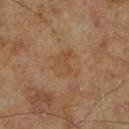The lesion was photographed on a routine skin check and not biopsied; there is no pathology result. Located on the right lower leg. Captured under cross-polarized illumination. The patient is a male about 70 years old. An algorithmic analysis of the crop reported a classifier nevus-likeness of about 0/100 and a detector confidence of about 100 out of 100 that the crop contains a lesion. A lesion tile, about 15 mm wide, cut from a 3D total-body photograph.